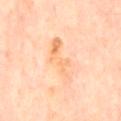Part of a total-body skin-imaging series; this lesion was reviewed on a skin check and was not flagged for biopsy. The tile uses cross-polarized illumination. Approximately 6.5 mm at its widest. A male patient aged around 65. The lesion is located on the mid back. A 15 mm crop from a total-body photograph taken for skin-cancer surveillance.